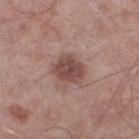biopsy_status: not biopsied; imaged during a skin examination
automated_metrics:
  area_mm2_approx: 9.0
  cielab_L: 47
  cielab_a: 20
  cielab_b: 22
  vs_skin_darker_L: 12.0
  vs_skin_contrast_norm: 8.5
  nevus_likeness_0_100: 45
  lesion_detection_confidence_0_100: 100
patient:
  sex: male
  age_approx: 55
image:
  source: total-body photography crop
  field_of_view_mm: 15
lesion_size:
  long_diameter_mm_approx: 3.5
lighting: white-light
site: left lower leg